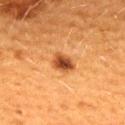{"biopsy_status": "not biopsied; imaged during a skin examination", "image": {"source": "total-body photography crop", "field_of_view_mm": 15}, "lesion_size": {"long_diameter_mm_approx": 3.0}, "lighting": "cross-polarized", "patient": {"sex": "female", "age_approx": 40}, "site": "back"}